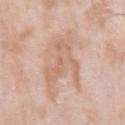notes: imaged on a skin check; not biopsied | TBP lesion metrics: an area of roughly 12 mm², an outline eccentricity of about 0.75 (0 = round, 1 = elongated), and a symmetry-axis asymmetry near 0.75; border irregularity of about 10 on a 0–10 scale, a within-lesion color-variation index near 2.5/10, and peripheral color asymmetry of about 0.5; an automated nevus-likeness rating near 0 out of 100 and a detector confidence of about 100 out of 100 that the crop contains a lesion | body site: the left upper arm | patient: male, aged approximately 75 | lesion diameter: ≈5.5 mm | acquisition: total-body-photography crop, ~15 mm field of view | tile lighting: white-light.